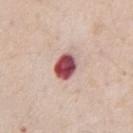<record>
  <patient>
    <sex>male</sex>
    <age_approx>70</age_approx>
  </patient>
  <lesion_size>
    <long_diameter_mm_approx>3.5</long_diameter_mm_approx>
  </lesion_size>
  <site>chest</site>
  <lighting>white-light</lighting>
  <image>
    <source>total-body photography crop</source>
    <field_of_view_mm>15</field_of_view_mm>
  </image>
</record>